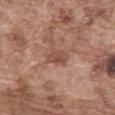follow-up — total-body-photography surveillance lesion; no biopsy | location — the abdomen | patient — male, in their mid-70s | size — ~2.5 mm (longest diameter) | lighting — white-light | imaging modality — total-body-photography crop, ~15 mm field of view.